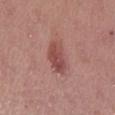Assessment: This lesion was catalogued during total-body skin photography and was not selected for biopsy. Context: This is a white-light tile. Measured at roughly 4 mm in maximum diameter. A female patient, aged 38 to 42. On the right lower leg. A roughly 15 mm field-of-view crop from a total-body skin photograph.